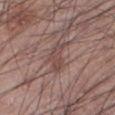Impression: Imaged during a routine full-body skin examination; the lesion was not biopsied and no histopathology is available. Clinical summary: The lesion is located on the left lower leg. The total-body-photography lesion software estimated a shape eccentricity near 0.85 and a symmetry-axis asymmetry near 0.45. The analysis additionally found a border-irregularity index near 5/10, a within-lesion color-variation index near 2.5/10, and a peripheral color-asymmetry measure near 1. It also reported an automated nevus-likeness rating near 0 out of 100 and a lesion-detection confidence of about 95/100. Captured under white-light illumination. A male subject in their 60s. The lesion's longest dimension is about 3.5 mm. A 15 mm close-up extracted from a 3D total-body photography capture.